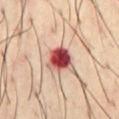Clinical impression:
Captured during whole-body skin photography for melanoma surveillance; the lesion was not biopsied.
Context:
The lesion is on the chest. The subject is a male roughly 40 years of age. Imaged with cross-polarized lighting. Automated image analysis of the tile measured an automated nevus-likeness rating near 0 out of 100 and lesion-presence confidence of about 100/100. A roughly 15 mm field-of-view crop from a total-body skin photograph.